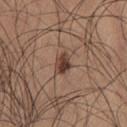This lesion was catalogued during total-body skin photography and was not selected for biopsy.
A male subject in their mid-60s.
A roughly 15 mm field-of-view crop from a total-body skin photograph.
Automated image analysis of the tile measured an area of roughly 4 mm², an outline eccentricity of about 0.7 (0 = round, 1 = elongated), and two-axis asymmetry of about 0.2. The analysis additionally found a classifier nevus-likeness of about 85/100 and lesion-presence confidence of about 100/100.
Measured at roughly 2.5 mm in maximum diameter.
Located on the left thigh.